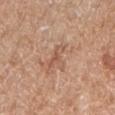No biopsy was performed on this lesion — it was imaged during a full skin examination and was not determined to be concerning.
A female patient, approximately 75 years of age.
Cropped from a whole-body photographic skin survey; the tile spans about 15 mm.
Imaged with white-light lighting.
About 3.5 mm across.
An algorithmic analysis of the crop reported an average lesion color of about L≈56 a*≈21 b*≈31 (CIELAB), a lesion–skin lightness drop of about 8, and a lesion-to-skin contrast of about 5.5 (normalized; higher = more distinct). And it measured border irregularity of about 7 on a 0–10 scale, internal color variation of about 0 on a 0–10 scale, and peripheral color asymmetry of about 0. And it measured a nevus-likeness score of about 0/100 and a lesion-detection confidence of about 95/100.
The lesion is located on the right forearm.